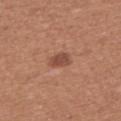biopsy status = imaged on a skin check; not biopsied | location = the right thigh | patient = female, aged 43 to 47 | imaging modality = ~15 mm crop, total-body skin-cancer survey.